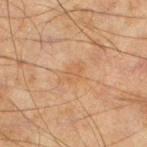notes — catalogued during a skin exam; not biopsied | site — the left lower leg | subject — male, aged 43–47 | size — about 3 mm | acquisition — ~15 mm tile from a whole-body skin photo | illumination — cross-polarized illumination.